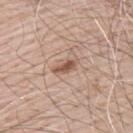• biopsy status — imaged on a skin check; not biopsied
• lighting — white-light illumination
• body site — the upper back
• lesion size — ~3 mm (longest diameter)
• subject — male, approximately 70 years of age
• imaging modality — ~15 mm tile from a whole-body skin photo